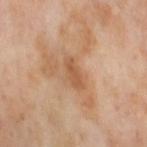Q: Was this lesion biopsied?
A: imaged on a skin check; not biopsied
Q: Lesion size?
A: about 3.5 mm
Q: What lighting was used for the tile?
A: cross-polarized
Q: Where on the body is the lesion?
A: the right thigh
Q: Who is the patient?
A: female, aged around 55
Q: What kind of image is this?
A: 15 mm crop, total-body photography